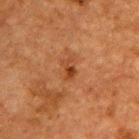Imaged during a routine full-body skin examination; the lesion was not biopsied and no histopathology is available.
A male patient, about 50 years old.
Located on the upper back.
A close-up tile cropped from a whole-body skin photograph, about 15 mm across.
The lesion's longest dimension is about 2.5 mm.